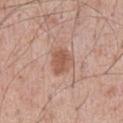The lesion was tiled from a total-body skin photograph and was not biopsied.